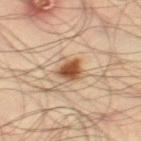Notes:
• workup · catalogued during a skin exam; not biopsied
• subject · male, roughly 35 years of age
• location · the leg
• acquisition · ~15 mm crop, total-body skin-cancer survey
• diameter · ≈3 mm